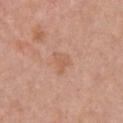Measured at roughly 3 mm in maximum diameter. A roughly 15 mm field-of-view crop from a total-body skin photograph. The lesion is on the chest. A male patient, aged 63 to 67.